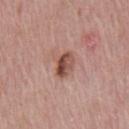Assessment: This lesion was catalogued during total-body skin photography and was not selected for biopsy. Image and clinical context: A male patient, roughly 70 years of age. A 15 mm close-up tile from a total-body photography series done for melanoma screening. The lesion-visualizer software estimated a shape eccentricity near 0.75 and two-axis asymmetry of about 0.15. Located on the chest. Captured under white-light illumination. The recorded lesion diameter is about 3.5 mm.